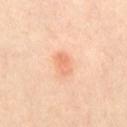Assessment: Imaged during a routine full-body skin examination; the lesion was not biopsied and no histopathology is available. Context: Automated image analysis of the tile measured an automated nevus-likeness rating near 85 out of 100 and lesion-presence confidence of about 100/100. From the abdomen. A female subject approximately 55 years of age. A region of skin cropped from a whole-body photographic capture, roughly 15 mm wide.